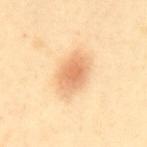The lesion was tiled from a total-body skin photograph and was not biopsied.
A female patient, approximately 40 years of age.
A region of skin cropped from a whole-body photographic capture, roughly 15 mm wide.
The lesion is located on the mid back.
The lesion's longest dimension is about 4.5 mm.